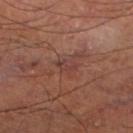notes = no biopsy performed (imaged during a skin exam) | subject = male, in their mid-60s | acquisition = total-body-photography crop, ~15 mm field of view | diameter = about 2.5 mm | illumination = cross-polarized illumination | anatomic site = the left lower leg | automated lesion analysis = a shape eccentricity near 0.9; a border-irregularity index near 5/10, a within-lesion color-variation index near 0/10, and radial color variation of about 0.